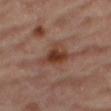<case>
  <biopsy_status>not biopsied; imaged during a skin examination</biopsy_status>
  <site>right thigh</site>
  <patient>
    <sex>male</sex>
    <age_approx>85+</age_approx>
  </patient>
  <lesion_size>
    <long_diameter_mm_approx>4.0</long_diameter_mm_approx>
  </lesion_size>
  <lighting>cross-polarized</lighting>
  <image>
    <source>total-body photography crop</source>
    <field_of_view_mm>15</field_of_view_mm>
  </image>
</case>